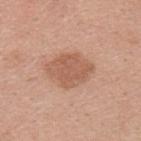Assessment:
The lesion was photographed on a routine skin check and not biopsied; there is no pathology result.
Clinical summary:
A male patient, in their mid-20s. A 15 mm close-up tile from a total-body photography series done for melanoma screening. The lesion's longest dimension is about 5 mm. The total-body-photography lesion software estimated a border-irregularity index near 2/10 and radial color variation of about 0.5. Imaged with white-light lighting. The lesion is located on the upper back.